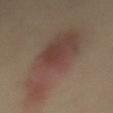Captured during whole-body skin photography for melanoma surveillance; the lesion was not biopsied. The subject is a female about 40 years old. Located on the back. This image is a 15 mm lesion crop taken from a total-body photograph. Captured under cross-polarized illumination. Measured at roughly 13 mm in maximum diameter.